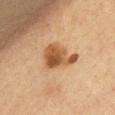Imaged during a routine full-body skin examination; the lesion was not biopsied and no histopathology is available. Longest diameter approximately 5 mm. Automated image analysis of the tile measured a classifier nevus-likeness of about 95/100 and lesion-presence confidence of about 100/100. The lesion is on the chest. A female subject approximately 50 years of age. Captured under cross-polarized illumination. A lesion tile, about 15 mm wide, cut from a 3D total-body photograph.